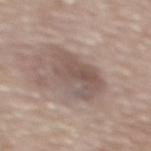Recorded during total-body skin imaging; not selected for excision or biopsy.
Located on the upper back.
Approximately 5.5 mm at its widest.
The subject is a male in their mid- to late 80s or older.
A 15 mm close-up tile from a total-body photography series done for melanoma screening.